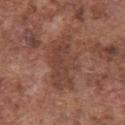<record>
  <biopsy_status>not biopsied; imaged during a skin examination</biopsy_status>
  <patient>
    <sex>male</sex>
    <age_approx>75</age_approx>
  </patient>
  <lighting>white-light</lighting>
  <image>
    <source>total-body photography crop</source>
    <field_of_view_mm>15</field_of_view_mm>
  </image>
  <site>chest</site>
  <lesion_size>
    <long_diameter_mm_approx>6.5</long_diameter_mm_approx>
  </lesion_size>
</record>